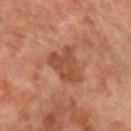Clinical impression:
This lesion was catalogued during total-body skin photography and was not selected for biopsy.
Context:
About 4 mm across. A region of skin cropped from a whole-body photographic capture, roughly 15 mm wide. The subject is a female about 65 years old. The tile uses cross-polarized illumination. Automated image analysis of the tile measured an area of roughly 11 mm², an outline eccentricity of about 0.35 (0 = round, 1 = elongated), and a shape-asymmetry score of about 0.3 (0 = symmetric). The lesion is located on the left forearm.